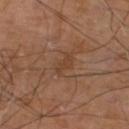| feature | finding |
|---|---|
| tile lighting | cross-polarized illumination |
| image | 15 mm crop, total-body photography |
| patient | male, aged 68 to 72 |
| automated lesion analysis | a footprint of about 3 mm² and a shape-asymmetry score of about 0.35 (0 = symmetric); an average lesion color of about L≈39 a*≈19 b*≈30 (CIELAB) and a normalized lesion–skin contrast near 5.5 |
| lesion diameter | about 2.5 mm |
| site | the left lower leg |